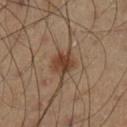Part of a total-body skin-imaging series; this lesion was reviewed on a skin check and was not flagged for biopsy. Imaged with cross-polarized lighting. The patient is a male roughly 40 years of age. Located on the left lower leg. About 3.5 mm across. An algorithmic analysis of the crop reported an area of roughly 8 mm² and an eccentricity of roughly 0.15. The analysis additionally found a normalized border contrast of about 8.5. It also reported a nevus-likeness score of about 90/100 and a detector confidence of about 100 out of 100 that the crop contains a lesion. A close-up tile cropped from a whole-body skin photograph, about 15 mm across.